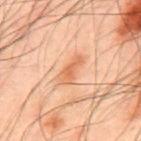Q: Was a biopsy performed?
A: imaged on a skin check; not biopsied
Q: Patient demographics?
A: male, aged approximately 50
Q: What did automated image analysis measure?
A: a border-irregularity rating of about 3.5/10, internal color variation of about 3 on a 0–10 scale, and a peripheral color-asymmetry measure near 0.5; lesion-presence confidence of about 100/100
Q: What kind of image is this?
A: 15 mm crop, total-body photography
Q: Where on the body is the lesion?
A: the upper back